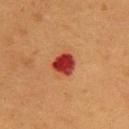Captured during whole-body skin photography for melanoma surveillance; the lesion was not biopsied. The tile uses cross-polarized illumination. The lesion is on the back. A male subject aged 53–57. This image is a 15 mm lesion crop taken from a total-body photograph.